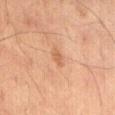follow-up=no biopsy performed (imaged during a skin exam)
patient=male, aged approximately 60
image=15 mm crop, total-body photography
lighting=cross-polarized
lesion size=≈2.5 mm
TBP lesion metrics=an automated nevus-likeness rating near 0 out of 100
body site=the abdomen